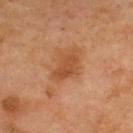Clinical impression:
Part of a total-body skin-imaging series; this lesion was reviewed on a skin check and was not flagged for biopsy.
Clinical summary:
Measured at roughly 4 mm in maximum diameter. A male patient, aged around 70. A roughly 15 mm field-of-view crop from a total-body skin photograph. On the upper back. This is a cross-polarized tile.